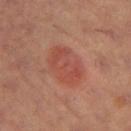Background:
The lesion is on the left thigh. A female patient, aged 38 to 42. Cropped from a total-body skin-imaging series; the visible field is about 15 mm.